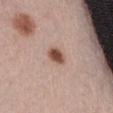Clinical impression:
This lesion was catalogued during total-body skin photography and was not selected for biopsy.
Context:
The total-body-photography lesion software estimated an area of roughly 4.5 mm², an eccentricity of roughly 0.45, and two-axis asymmetry of about 0.25. The analysis additionally found an average lesion color of about L≈51 a*≈21 b*≈27 (CIELAB), about 15 CIELAB-L* units darker than the surrounding skin, and a normalized border contrast of about 10.5. It also reported a classifier nevus-likeness of about 100/100 and a lesion-detection confidence of about 100/100. About 2.5 mm across. On the abdomen. A female subject, in their mid- to late 60s. A roughly 15 mm field-of-view crop from a total-body skin photograph.